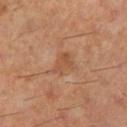• follow-up: total-body-photography surveillance lesion; no biopsy
• patient: male, roughly 60 years of age
• site: the left lower leg
• imaging modality: ~15 mm crop, total-body skin-cancer survey